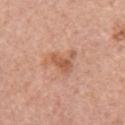biopsy status: catalogued during a skin exam; not biopsied